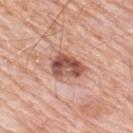Q: Is there a histopathology result?
A: imaged on a skin check; not biopsied
Q: What are the patient's age and sex?
A: male, aged around 80
Q: Lesion size?
A: about 4.5 mm
Q: What is the imaging modality?
A: ~15 mm tile from a whole-body skin photo
Q: Lesion location?
A: the upper back
Q: Illumination type?
A: white-light